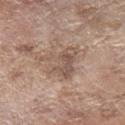Notes:
- image: ~15 mm crop, total-body skin-cancer survey
- diameter: about 5 mm
- subject: female, about 75 years old
- automated metrics: a mean CIELAB color near L≈53 a*≈16 b*≈25 and roughly 9 lightness units darker than nearby skin; a nevus-likeness score of about 5/100
- anatomic site: the right forearm
- lighting: white-light